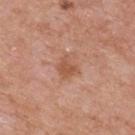{"biopsy_status": "not biopsied; imaged during a skin examination", "automated_metrics": {"area_mm2_approx": 4.5, "eccentricity": 0.5, "shape_asymmetry": 0.3, "vs_skin_darker_L": 8.0, "vs_skin_contrast_norm": 6.5, "border_irregularity_0_10": 3.0, "peripheral_color_asymmetry": 0.5, "nevus_likeness_0_100": 5}, "lesion_size": {"long_diameter_mm_approx": 2.5}, "patient": {"sex": "male", "age_approx": 75}, "image": {"source": "total-body photography crop", "field_of_view_mm": 15}, "site": "back"}